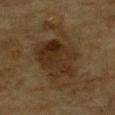Clinical impression: This lesion was catalogued during total-body skin photography and was not selected for biopsy. Acquisition and patient details: The lesion is located on the chest. A male subject, aged around 85 or older. The lesion's longest dimension is about 8.5 mm. A close-up tile cropped from a whole-body skin photograph, about 15 mm across.